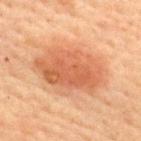Clinical summary:
Measured at roughly 8 mm in maximum diameter. A roughly 15 mm field-of-view crop from a total-body skin photograph. The lesion-visualizer software estimated a footprint of about 30 mm² and a shape-asymmetry score of about 0.15 (0 = symmetric). And it measured a border-irregularity index near 2/10, a within-lesion color-variation index near 4/10, and radial color variation of about 1.5. Imaged with cross-polarized lighting. The lesion is located on the upper back. The subject is a male aged around 60.